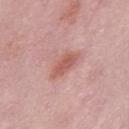Assessment: Part of a total-body skin-imaging series; this lesion was reviewed on a skin check and was not flagged for biopsy. Context: The total-body-photography lesion software estimated an average lesion color of about L≈58 a*≈26 b*≈26 (CIELAB), roughly 10 lightness units darker than nearby skin, and a normalized border contrast of about 7. And it measured a border-irregularity rating of about 2.5/10 and internal color variation of about 2 on a 0–10 scale. Measured at roughly 4 mm in maximum diameter. A male subject approximately 55 years of age. This image is a 15 mm lesion crop taken from a total-body photograph. The lesion is located on the back.